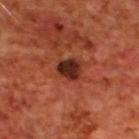Q: Was a biopsy performed?
A: total-body-photography surveillance lesion; no biopsy
Q: What kind of image is this?
A: total-body-photography crop, ~15 mm field of view
Q: Automated lesion metrics?
A: a mean CIELAB color near L≈28 a*≈26 b*≈27, about 14 CIELAB-L* units darker than the surrounding skin, and a normalized border contrast of about 12.5; border irregularity of about 2 on a 0–10 scale, a color-variation rating of about 4.5/10, and peripheral color asymmetry of about 1.5
Q: What is the lesion's diameter?
A: ~3 mm (longest diameter)
Q: Where on the body is the lesion?
A: the upper back
Q: How was the tile lit?
A: cross-polarized
Q: What are the patient's age and sex?
A: male, in their 70s